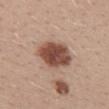<case>
  <site>mid back</site>
  <automated_metrics>
    <border_irregularity_0_10>2.0</border_irregularity_0_10>
    <color_variation_0_10>4.5</color_variation_0_10>
    <nevus_likeness_0_100>100</nevus_likeness_0_100>
    <lesion_detection_confidence_0_100>100</lesion_detection_confidence_0_100>
  </automated_metrics>
  <patient>
    <sex>female</sex>
    <age_approx>30</age_approx>
  </patient>
  <lighting>white-light</lighting>
  <image>
    <source>total-body photography crop</source>
    <field_of_view_mm>15</field_of_view_mm>
  </image>
</case>